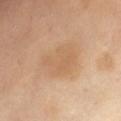Notes:
* site — the abdomen
* patient — female, aged approximately 55
* size — about 5 mm
* imaging modality — total-body-photography crop, ~15 mm field of view
* tile lighting — cross-polarized